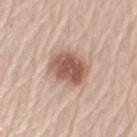The subject is a male approximately 70 years of age.
A region of skin cropped from a whole-body photographic capture, roughly 15 mm wide.
From the left upper arm.
The tile uses white-light illumination.
An algorithmic analysis of the crop reported a mean CIELAB color near L≈56 a*≈21 b*≈26, about 15 CIELAB-L* units darker than the surrounding skin, and a lesion-to-skin contrast of about 9.5 (normalized; higher = more distinct). And it measured a lesion-detection confidence of about 100/100.
About 4.5 mm across.